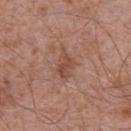Q: Is there a histopathology result?
A: no biopsy performed (imaged during a skin exam)
Q: Lesion location?
A: the abdomen
Q: Patient demographics?
A: male, in their 70s
Q: What kind of image is this?
A: ~15 mm crop, total-body skin-cancer survey